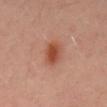Image and clinical context:
The patient is a male roughly 55 years of age. Located on the mid back. A roughly 15 mm field-of-view crop from a total-body skin photograph. The lesion's longest dimension is about 3.5 mm. Captured under cross-polarized illumination.